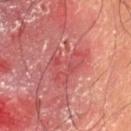biopsy status: catalogued during a skin exam; not biopsied
lighting: cross-polarized illumination
lesion size: ~5 mm (longest diameter)
patient: male, approximately 55 years of age
TBP lesion metrics: a footprint of about 12 mm², an outline eccentricity of about 0.65 (0 = round, 1 = elongated), and two-axis asymmetry of about 0.5; a mean CIELAB color near L≈46 a*≈32 b*≈23, a lesion–skin lightness drop of about 6, and a normalized border contrast of about 5
body site: the left thigh
acquisition: ~15 mm tile from a whole-body skin photo